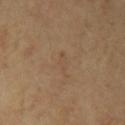{
  "biopsy_status": "not biopsied; imaged during a skin examination",
  "lesion_size": {
    "long_diameter_mm_approx": 2.5
  },
  "automated_metrics": {
    "area_mm2_approx": 2.5,
    "eccentricity": 0.9,
    "cielab_L": 48,
    "cielab_a": 15,
    "cielab_b": 30,
    "vs_skin_darker_L": 4.0,
    "color_variation_0_10": 0.0,
    "peripheral_color_asymmetry": 0.0
  },
  "lighting": "cross-polarized",
  "image": {
    "source": "total-body photography crop",
    "field_of_view_mm": 15
  },
  "site": "right upper arm",
  "patient": {
    "sex": "male",
    "age_approx": 55
  }
}